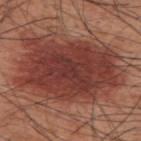  biopsy_status: not biopsied; imaged during a skin examination
  image:
    source: total-body photography crop
    field_of_view_mm: 15
  patient:
    sex: male
    age_approx: 60
  lighting: white-light
  site: back
  lesion_size:
    long_diameter_mm_approx: 11.0